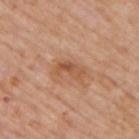workup — no biopsy performed (imaged during a skin exam) | lesion size — ~3.5 mm (longest diameter) | patient — male, approximately 75 years of age | acquisition — ~15 mm crop, total-body skin-cancer survey | image-analysis metrics — a footprint of about 4.5 mm²; an average lesion color of about L≈54 a*≈23 b*≈35 (CIELAB) and roughly 9 lightness units darker than nearby skin; a classifier nevus-likeness of about 20/100 and lesion-presence confidence of about 100/100 | tile lighting — white-light | body site — the upper back.